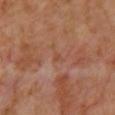Notes:
• workup — imaged on a skin check; not biopsied
• image source — 15 mm crop, total-body photography
• patient — female
• anatomic site — the upper back